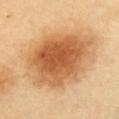biopsy status: imaged on a skin check; not biopsied
acquisition: 15 mm crop, total-body photography
anatomic site: the chest
subject: female, in their 60s